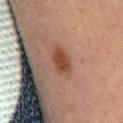| feature | finding |
|---|---|
| follow-up | imaged on a skin check; not biopsied |
| patient | male, about 65 years old |
| image | 15 mm crop, total-body photography |
| lighting | cross-polarized illumination |
| site | the abdomen |
| automated lesion analysis | an automated nevus-likeness rating near 100 out of 100 and lesion-presence confidence of about 100/100 |